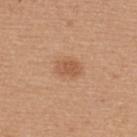<tbp_lesion>
  <biopsy_status>not biopsied; imaged during a skin examination</biopsy_status>
  <image>
    <source>total-body photography crop</source>
    <field_of_view_mm>15</field_of_view_mm>
  </image>
  <patient>
    <sex>male</sex>
    <age_approx>40</age_approx>
  </patient>
  <lesion_size>
    <long_diameter_mm_approx>2.5</long_diameter_mm_approx>
  </lesion_size>
  <site>back</site>
</tbp_lesion>